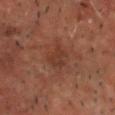* notes · imaged on a skin check; not biopsied
* image-analysis metrics · an eccentricity of roughly 0.55; about 5 CIELAB-L* units darker than the surrounding skin and a normalized lesion–skin contrast near 5.5; an automated nevus-likeness rating near 0 out of 100 and a lesion-detection confidence of about 100/100
* body site · the head or neck
* size · ~3 mm (longest diameter)
* image source · ~15 mm tile from a whole-body skin photo
* patient · male, aged 48–52
* lighting · cross-polarized